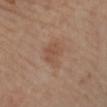Clinical impression:
Part of a total-body skin-imaging series; this lesion was reviewed on a skin check and was not flagged for biopsy.
Background:
Cropped from a whole-body photographic skin survey; the tile spans about 15 mm. Located on the left lower leg. Longest diameter approximately 3 mm. A female subject aged 48–52.